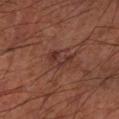  site: left forearm
  lesion_size:
    long_diameter_mm_approx: 3.5
  image:
    source: total-body photography crop
    field_of_view_mm: 15
  lighting: cross-polarized
  patient:
    sex: male
    age_approx: 60
  automated_metrics:
    area_mm2_approx: 5.5
    eccentricity: 0.75
    shape_asymmetry: 0.45
    border_irregularity_0_10: 4.5
    color_variation_0_10: 4.5
    peripheral_color_asymmetry: 1.5